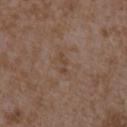Captured during whole-body skin photography for melanoma surveillance; the lesion was not biopsied. Cropped from a total-body skin-imaging series; the visible field is about 15 mm. A female patient about 35 years old. From the right forearm.